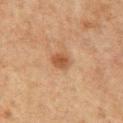Findings:
- workup · catalogued during a skin exam; not biopsied